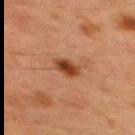The lesion was tiled from a total-body skin photograph and was not biopsied. From the upper back. A lesion tile, about 15 mm wide, cut from a 3D total-body photograph. The subject is a male approximately 60 years of age. Imaged with cross-polarized lighting. The recorded lesion diameter is about 3 mm.